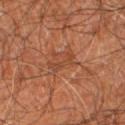Clinical impression: Recorded during total-body skin imaging; not selected for excision or biopsy. Context: On the right leg. A male patient, aged 58–62. A region of skin cropped from a whole-body photographic capture, roughly 15 mm wide.